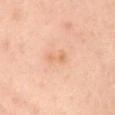Clinical summary:
Cropped from a whole-body photographic skin survey; the tile spans about 15 mm. This is a cross-polarized tile. A male patient aged approximately 40. The lesion is on the mid back.